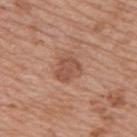Cropped from a total-body skin-imaging series; the visible field is about 15 mm.
The recorded lesion diameter is about 3.5 mm.
Imaged with white-light lighting.
A female subject, aged 43–47.
The lesion is on the back.